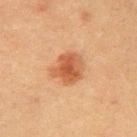Recorded during total-body skin imaging; not selected for excision or biopsy.
Imaged with cross-polarized lighting.
The subject is a male aged 38 to 42.
Cropped from a total-body skin-imaging series; the visible field is about 15 mm.
About 4 mm across.
The lesion is located on the arm.